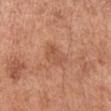No biopsy was performed on this lesion — it was imaged during a full skin examination and was not determined to be concerning. Automated tile analysis of the lesion measured an average lesion color of about L≈54 a*≈24 b*≈33 (CIELAB), about 7 CIELAB-L* units darker than the surrounding skin, and a normalized border contrast of about 5. And it measured a nevus-likeness score of about 5/100. On the right upper arm. The patient is a male roughly 65 years of age. A 15 mm close-up tile from a total-body photography series done for melanoma screening. This is a white-light tile.